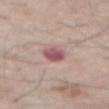No biopsy was performed on this lesion — it was imaged during a full skin examination and was not determined to be concerning. A male subject about 80 years old. On the abdomen. A 15 mm close-up extracted from a 3D total-body photography capture.